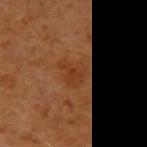Q: Is there a histopathology result?
A: total-body-photography surveillance lesion; no biopsy
Q: What are the patient's age and sex?
A: male, aged 58–62
Q: How was this image acquired?
A: 15 mm crop, total-body photography
Q: Illumination type?
A: cross-polarized illumination
Q: Where on the body is the lesion?
A: the right upper arm
Q: What is the lesion's diameter?
A: ≈3.5 mm